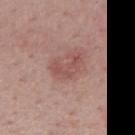Clinical impression: The lesion was tiled from a total-body skin photograph and was not biopsied. Clinical summary: A 15 mm close-up tile from a total-body photography series done for melanoma screening. A male patient approximately 50 years of age. The lesion-visualizer software estimated an average lesion color of about L≈51 a*≈23 b*≈24 (CIELAB) and roughly 8 lightness units darker than nearby skin. The software also gave a within-lesion color-variation index near 2/10 and radial color variation of about 0.5. The lesion is on the mid back.